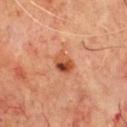Imaged during a routine full-body skin examination; the lesion was not biopsied and no histopathology is available.
A male subject, approximately 70 years of age.
A lesion tile, about 15 mm wide, cut from a 3D total-body photograph.
From the front of the torso.
Imaged with cross-polarized lighting.
Automated tile analysis of the lesion measured a lesion area of about 5 mm² and an outline eccentricity of about 0.7 (0 = round, 1 = elongated). The analysis additionally found border irregularity of about 1.5 on a 0–10 scale, a within-lesion color-variation index near 10/10, and peripheral color asymmetry of about 4.5.